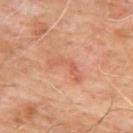Recorded during total-body skin imaging; not selected for excision or biopsy. This image is a 15 mm lesion crop taken from a total-body photograph. The lesion is located on the upper back. The patient is a male in their mid- to late 60s.